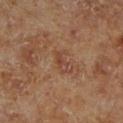The lesion was tiled from a total-body skin photograph and was not biopsied.